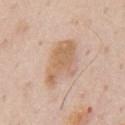Case summary:
- patient: male, aged around 60
- acquisition: ~15 mm tile from a whole-body skin photo
- site: the chest